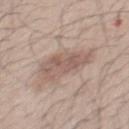Q: Was this lesion biopsied?
A: no biopsy performed (imaged during a skin exam)
Q: Lesion size?
A: about 6 mm
Q: How was the tile lit?
A: white-light
Q: What kind of image is this?
A: ~15 mm tile from a whole-body skin photo
Q: What did automated image analysis measure?
A: an area of roughly 13 mm², an outline eccentricity of about 0.85 (0 = round, 1 = elongated), and a shape-asymmetry score of about 0.3 (0 = symmetric); an average lesion color of about L≈57 a*≈16 b*≈24 (CIELAB), about 9 CIELAB-L* units darker than the surrounding skin, and a normalized lesion–skin contrast near 6.5
Q: Who is the patient?
A: male, aged 38 to 42
Q: Where on the body is the lesion?
A: the mid back